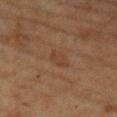Findings:
* biopsy status: total-body-photography surveillance lesion; no biopsy
* lesion diameter: about 3 mm
* illumination: cross-polarized illumination
* body site: the right forearm
* acquisition: 15 mm crop, total-body photography
* image-analysis metrics: a lesion color around L≈35 a*≈17 b*≈26 in CIELAB; border irregularity of about 3 on a 0–10 scale and peripheral color asymmetry of about 0.5; an automated nevus-likeness rating near 0 out of 100 and a detector confidence of about 100 out of 100 that the crop contains a lesion
* subject: female, about 80 years old